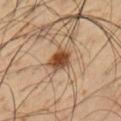This lesion was catalogued during total-body skin photography and was not selected for biopsy.